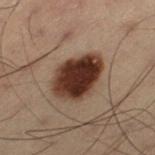image-analysis metrics — a lesion area of about 19 mm², an outline eccentricity of about 0.65 (0 = round, 1 = elongated), and two-axis asymmetry of about 0.15; roughly 17 lightness units darker than nearby skin and a normalized lesion–skin contrast near 16; a border-irregularity rating of about 1.5/10, internal color variation of about 5 on a 0–10 scale, and radial color variation of about 1.5
image — ~15 mm tile from a whole-body skin photo
diameter — ~5.5 mm (longest diameter)
subject — male, aged around 55
body site — the left thigh